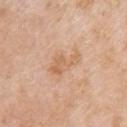<case>
<biopsy_status>not biopsied; imaged during a skin examination</biopsy_status>
<site>right upper arm</site>
<image>
  <source>total-body photography crop</source>
  <field_of_view_mm>15</field_of_view_mm>
</image>
<patient>
  <sex>female</sex>
  <age_approx>70</age_approx>
</patient>
</case>